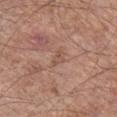biopsy_status: not biopsied; imaged during a skin examination
patient:
  sex: male
  age_approx: 60
automated_metrics:
  cielab_L: 53
  cielab_a: 20
  cielab_b: 27
  vs_skin_darker_L: 6.0
  vs_skin_contrast_norm: 5.0
  nevus_likeness_0_100: 0
  lesion_detection_confidence_0_100: 100
site: right lower leg
image:
  source: total-body photography crop
  field_of_view_mm: 15